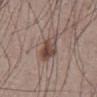The lesion was photographed on a routine skin check and not biopsied; there is no pathology result. A close-up tile cropped from a whole-body skin photograph, about 15 mm across. The tile uses white-light illumination. On the abdomen. The subject is a male in their mid- to late 50s. Automated tile analysis of the lesion measured a lesion area of about 9 mm², an outline eccentricity of about 0.85 (0 = round, 1 = elongated), and two-axis asymmetry of about 0.25. It also reported a lesion color around L≈46 a*≈16 b*≈22 in CIELAB, about 11 CIELAB-L* units darker than the surrounding skin, and a normalized border contrast of about 8.5. It also reported a classifier nevus-likeness of about 85/100 and a lesion-detection confidence of about 100/100.